Cropped from a whole-body photographic skin survey; the tile spans about 15 mm; the patient is a female roughly 40 years of age; the lesion is on the right upper arm:
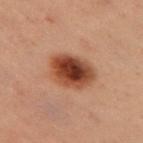Notes:
– diameter — ≈4.5 mm
– illumination — cross-polarized illumination
– diagnosis — a dysplastic (Clark) nevus